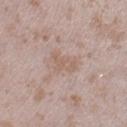Assessment:
Captured during whole-body skin photography for melanoma surveillance; the lesion was not biopsied.
Context:
The lesion is located on the right lower leg. A roughly 15 mm field-of-view crop from a total-body skin photograph. A female patient, aged 23 to 27. The recorded lesion diameter is about 3.5 mm.